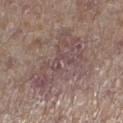Clinical impression: No biopsy was performed on this lesion — it was imaged during a full skin examination and was not determined to be concerning. Clinical summary: Captured under white-light illumination. Automated tile analysis of the lesion measured a shape-asymmetry score of about 0.45 (0 = symmetric). The analysis additionally found roughly 7 lightness units darker than nearby skin. The analysis additionally found a classifier nevus-likeness of about 0/100. A 15 mm close-up extracted from a 3D total-body photography capture. Measured at roughly 8 mm in maximum diameter. From the left lower leg. The patient is a male in their mid-60s.